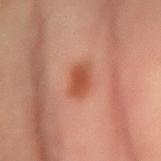body site — the right forearm | image source — total-body-photography crop, ~15 mm field of view | patient — male, aged 48–52.